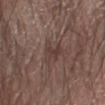<record>
<biopsy_status>not biopsied; imaged during a skin examination</biopsy_status>
<lighting>white-light</lighting>
<patient>
  <sex>male</sex>
  <age_approx>65</age_approx>
</patient>
<site>left forearm</site>
<image>
  <source>total-body photography crop</source>
  <field_of_view_mm>15</field_of_view_mm>
</image>
<lesion_size>
  <long_diameter_mm_approx>4.0</long_diameter_mm_approx>
</lesion_size>
</record>